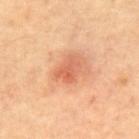image — ~15 mm tile from a whole-body skin photo | body site — the mid back | patient — male, in their 70s.